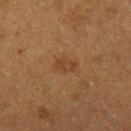Clinical impression:
Imaged during a routine full-body skin examination; the lesion was not biopsied and no histopathology is available.
Image and clinical context:
On the left forearm. A 15 mm close-up tile from a total-body photography series done for melanoma screening. A female patient, aged 38–42. Longest diameter approximately 3 mm.